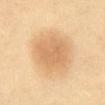Imaged during a routine full-body skin examination; the lesion was not biopsied and no histopathology is available.
The tile uses cross-polarized illumination.
A lesion tile, about 15 mm wide, cut from a 3D total-body photograph.
On the abdomen.
The subject is aged approximately 70.
Automated tile analysis of the lesion measured an area of roughly 14 mm², an eccentricity of roughly 0.5, and a symmetry-axis asymmetry near 0.15. The analysis additionally found an automated nevus-likeness rating near 95 out of 100.
The lesion's longest dimension is about 4.5 mm.